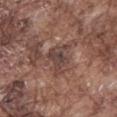Background: A male subject in their mid-70s. This is a white-light tile. A roughly 15 mm field-of-view crop from a total-body skin photograph. Located on the mid back. Measured at roughly 3.5 mm in maximum diameter.